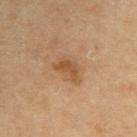Captured during whole-body skin photography for melanoma surveillance; the lesion was not biopsied. Approximately 4 mm at its widest. Imaged with cross-polarized lighting. The lesion is on the right upper arm. A lesion tile, about 15 mm wide, cut from a 3D total-body photograph. The subject is a male approximately 45 years of age. Automated tile analysis of the lesion measured a footprint of about 8 mm², an outline eccentricity of about 0.8 (0 = round, 1 = elongated), and a symmetry-axis asymmetry near 0.2. It also reported a lesion color around L≈45 a*≈17 b*≈31 in CIELAB, roughly 7 lightness units darker than nearby skin, and a normalized border contrast of about 6.5. It also reported lesion-presence confidence of about 100/100.